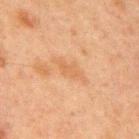No biopsy was performed on this lesion — it was imaged during a full skin examination and was not determined to be concerning. From the front of the torso. The recorded lesion diameter is about 4.5 mm. A roughly 15 mm field-of-view crop from a total-body skin photograph. This is a cross-polarized tile. The total-body-photography lesion software estimated a lesion color around L≈51 a*≈18 b*≈33 in CIELAB, a lesion–skin lightness drop of about 5, and a lesion-to-skin contrast of about 5 (normalized; higher = more distinct). The analysis additionally found a border-irregularity index near 4/10 and radial color variation of about 0.5. It also reported a nevus-likeness score of about 0/100. The patient is a male aged approximately 65.